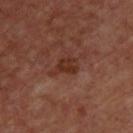Q: Was a biopsy performed?
A: total-body-photography surveillance lesion; no biopsy